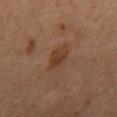Q: Was a biopsy performed?
A: imaged on a skin check; not biopsied
Q: What is the lesion's diameter?
A: ≈3.5 mm
Q: What are the patient's age and sex?
A: male, aged around 60
Q: Lesion location?
A: the front of the torso
Q: What kind of image is this?
A: ~15 mm crop, total-body skin-cancer survey
Q: Automated lesion metrics?
A: an area of roughly 5.5 mm² and a shape-asymmetry score of about 0.3 (0 = symmetric)